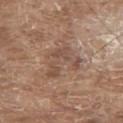Notes:
– workup: catalogued during a skin exam; not biopsied
– lesion size: ≈5 mm
– patient: male, about 80 years old
– imaging modality: ~15 mm tile from a whole-body skin photo
– location: the back